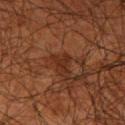image-analysis metrics — a lesion area of about 3 mm², a shape eccentricity near 0.55, and a symmetry-axis asymmetry near 0.65; a border-irregularity rating of about 7/10, a color-variation rating of about 0/10, and radial color variation of about 0; a classifier nevus-likeness of about 0/100 and lesion-presence confidence of about 95/100
lesion size — about 2.5 mm
illumination — cross-polarized illumination
image — total-body-photography crop, ~15 mm field of view
site — the right upper arm
subject — male, roughly 45 years of age